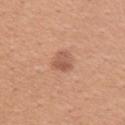{"biopsy_status": "not biopsied; imaged during a skin examination", "site": "right upper arm", "image": {"source": "total-body photography crop", "field_of_view_mm": 15}, "lesion_size": {"long_diameter_mm_approx": 3.0}, "lighting": "white-light", "patient": {"sex": "female", "age_approx": 40}}